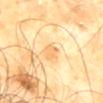Assessment:
The lesion was tiled from a total-body skin photograph and was not biopsied.
Clinical summary:
The patient is a male aged around 65. Cropped from a whole-body photographic skin survey; the tile spans about 15 mm. The lesion is on the mid back. The recorded lesion diameter is about 2.5 mm.